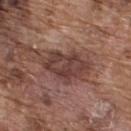biopsy status = imaged on a skin check; not biopsied
location = the back
image = 15 mm crop, total-body photography
subject = male, aged 73 to 77